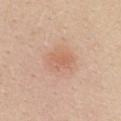A lesion tile, about 15 mm wide, cut from a 3D total-body photograph. Automated image analysis of the tile measured a lesion area of about 7 mm², an eccentricity of roughly 0.65, and two-axis asymmetry of about 0.15. The software also gave an average lesion color of about L≈63 a*≈22 b*≈31 (CIELAB), roughly 7 lightness units darker than nearby skin, and a lesion-to-skin contrast of about 5 (normalized; higher = more distinct). The analysis additionally found a border-irregularity rating of about 1.5/10 and a peripheral color-asymmetry measure near 1. From the mid back. The subject is a female approximately 20 years of age. Imaged with white-light lighting. The lesion's longest dimension is about 3.5 mm.